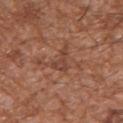Q: Was a biopsy performed?
A: imaged on a skin check; not biopsied
Q: Lesion size?
A: ≈3 mm
Q: What are the patient's age and sex?
A: male, in their mid- to late 40s
Q: What is the anatomic site?
A: the left upper arm
Q: What did automated image analysis measure?
A: a lesion area of about 4 mm², an eccentricity of roughly 0.85, and two-axis asymmetry of about 0.65; a lesion color around L≈43 a*≈23 b*≈29 in CIELAB, about 7 CIELAB-L* units darker than the surrounding skin, and a normalized lesion–skin contrast near 6; a border-irregularity rating of about 6.5/10 and a peripheral color-asymmetry measure near 0.5; a classifier nevus-likeness of about 0/100 and lesion-presence confidence of about 95/100
Q: What is the imaging modality?
A: ~15 mm crop, total-body skin-cancer survey
Q: What lighting was used for the tile?
A: white-light illumination